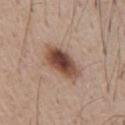biopsy status = imaged on a skin check; not biopsied | illumination = white-light illumination | size = ~5.5 mm (longest diameter) | image-analysis metrics = a shape eccentricity near 0.85; a within-lesion color-variation index near 8/10; a classifier nevus-likeness of about 95/100 and a lesion-detection confidence of about 100/100 | image source = ~15 mm crop, total-body skin-cancer survey | subject = male, aged approximately 55.